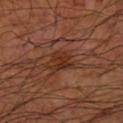workup: catalogued during a skin exam; not biopsied | TBP lesion metrics: a lesion area of about 5.5 mm²; an average lesion color of about L≈31 a*≈23 b*≈29 (CIELAB) and a lesion–skin lightness drop of about 8; a border-irregularity index near 4.5/10 and a color-variation rating of about 2/10 | patient: male, roughly 65 years of age | illumination: cross-polarized illumination | body site: the left upper arm | lesion size: ~3 mm (longest diameter) | imaging modality: ~15 mm crop, total-body skin-cancer survey.